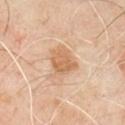biopsy_status: not biopsied; imaged during a skin examination
lesion_size:
  long_diameter_mm_approx: 4.0
site: chest
image:
  source: total-body photography crop
  field_of_view_mm: 15
patient:
  sex: male
  age_approx: 50